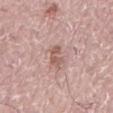Recorded during total-body skin imaging; not selected for excision or biopsy. This is a white-light tile. From the mid back. Longest diameter approximately 3.5 mm. A 15 mm crop from a total-body photograph taken for skin-cancer surveillance. The subject is a male aged 73–77.